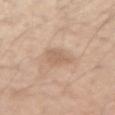Impression: No biopsy was performed on this lesion — it was imaged during a full skin examination and was not determined to be concerning. Acquisition and patient details: The tile uses white-light illumination. The lesion is on the right upper arm. The total-body-photography lesion software estimated a lesion area of about 5.5 mm² and a symmetry-axis asymmetry near 0.35. It also reported a color-variation rating of about 1.5/10 and a peripheral color-asymmetry measure near 0.5. The subject is a male in their 50s. A lesion tile, about 15 mm wide, cut from a 3D total-body photograph.